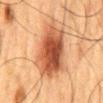biopsy_status: not biopsied; imaged during a skin examination
image:
  source: total-body photography crop
  field_of_view_mm: 15
automated_metrics:
  border_irregularity_0_10: 3.0
  color_variation_0_10: 7.5
  peripheral_color_asymmetry: 2.0
lighting: cross-polarized
lesion_size:
  long_diameter_mm_approx: 7.5
patient:
  sex: male
  age_approx: 60
site: mid back